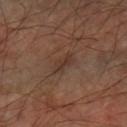• workup · catalogued during a skin exam; not biopsied
• body site · the right upper arm
• imaging modality · 15 mm crop, total-body photography
• patient · male, aged 58–62
• lighting · cross-polarized
• size · about 3.5 mm